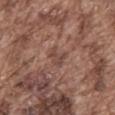Case summary:
• lighting: white-light illumination
• imaging modality: ~15 mm tile from a whole-body skin photo
• subject: male, approximately 75 years of age
• site: the mid back
• automated metrics: a symmetry-axis asymmetry near 0.4; an average lesion color of about L≈45 a*≈19 b*≈24 (CIELAB) and a lesion–skin lightness drop of about 7; internal color variation of about 1.5 on a 0–10 scale